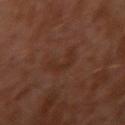workup: imaged on a skin check; not biopsied
illumination: cross-polarized
image-analysis metrics: a footprint of about 6.5 mm², an outline eccentricity of about 0.9 (0 = round, 1 = elongated), and a symmetry-axis asymmetry near 0.55; an average lesion color of about L≈29 a*≈19 b*≈25 (CIELAB), a lesion–skin lightness drop of about 4, and a lesion-to-skin contrast of about 4.5 (normalized; higher = more distinct); a border-irregularity rating of about 6.5/10, a color-variation rating of about 1.5/10, and radial color variation of about 0.5; an automated nevus-likeness rating near 0 out of 100 and a detector confidence of about 100 out of 100 that the crop contains a lesion
body site: the left arm
acquisition: 15 mm crop, total-body photography
diameter: ≈4.5 mm
patient: male, about 30 years old